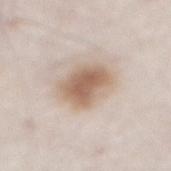Case summary:
- site: the abdomen
- image source: 15 mm crop, total-body photography
- subject: male, aged approximately 80